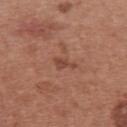| key | value |
|---|---|
| notes | no biopsy performed (imaged during a skin exam) |
| patient | female, roughly 40 years of age |
| anatomic site | the upper back |
| imaging modality | total-body-photography crop, ~15 mm field of view |